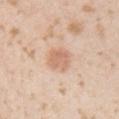Part of a total-body skin-imaging series; this lesion was reviewed on a skin check and was not flagged for biopsy. A male patient roughly 25 years of age. Automated image analysis of the tile measured a lesion area of about 6 mm² and a shape eccentricity near 0.6. And it measured a color-variation rating of about 2.5/10 and radial color variation of about 1. The analysis additionally found a classifier nevus-likeness of about 90/100 and a lesion-detection confidence of about 100/100. A roughly 15 mm field-of-view crop from a total-body skin photograph. Captured under white-light illumination. The recorded lesion diameter is about 3 mm. From the right upper arm.